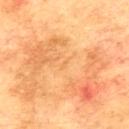| key | value |
|---|---|
| image | ~15 mm tile from a whole-body skin photo |
| lesion size | ≈1 mm |
| patient | male, aged 73 to 77 |
| TBP lesion metrics | a footprint of about 1 mm², an eccentricity of roughly 0.85, and a shape-asymmetry score of about 0.4 (0 = symmetric); a classifier nevus-likeness of about 0/100 and a lesion-detection confidence of about 100/100 |
| site | the upper back |
| lighting | cross-polarized |